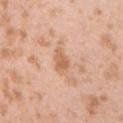This lesion was catalogued during total-body skin photography and was not selected for biopsy. A 15 mm crop from a total-body photograph taken for skin-cancer surveillance. Imaged with white-light lighting. The patient is a male about 25 years old. From the right upper arm. The total-body-photography lesion software estimated a border-irregularity rating of about 3/10, internal color variation of about 2.5 on a 0–10 scale, and radial color variation of about 1. The recorded lesion diameter is about 2.5 mm.